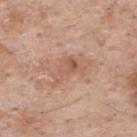workup: total-body-photography surveillance lesion; no biopsy
automated lesion analysis: a mean CIELAB color near L≈57 a*≈22 b*≈29, roughly 8 lightness units darker than nearby skin, and a lesion-to-skin contrast of about 5.5 (normalized; higher = more distinct); a classifier nevus-likeness of about 0/100 and a lesion-detection confidence of about 100/100
tile lighting: white-light
patient: male, aged 58 to 62
imaging modality: 15 mm crop, total-body photography
body site: the upper back